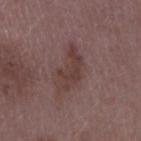The lesion is located on the right upper arm. Approximately 5 mm at its widest. A lesion tile, about 15 mm wide, cut from a 3D total-body photograph. The tile uses white-light illumination. A female patient aged 43–47.